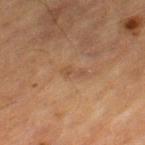The lesion was photographed on a routine skin check and not biopsied; there is no pathology result. Captured under cross-polarized illumination. About 2.5 mm across. A male subject approximately 85 years of age or older. A roughly 15 mm field-of-view crop from a total-body skin photograph. On the left thigh.